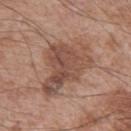Clinical impression: The lesion was photographed on a routine skin check and not biopsied; there is no pathology result. Context: The lesion is on the left upper arm. A 15 mm close-up tile from a total-body photography series done for melanoma screening. The tile uses white-light illumination. A male patient, roughly 65 years of age.